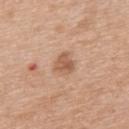biopsy status — imaged on a skin check; not biopsied | location — the upper back | patient — male, approximately 50 years of age | image source — 15 mm crop, total-body photography.